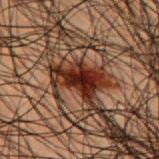No biopsy was performed on this lesion — it was imaged during a full skin examination and was not determined to be concerning. The lesion is on the upper back. A 15 mm crop from a total-body photograph taken for skin-cancer surveillance. Approximately 4.5 mm at its widest. A male patient, roughly 50 years of age.